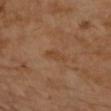Clinical impression:
This lesion was catalogued during total-body skin photography and was not selected for biopsy.
Clinical summary:
The patient is a female in their 70s. Cropped from a whole-body photographic skin survey; the tile spans about 15 mm. The lesion is located on the arm.